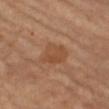workup: catalogued during a skin exam; not biopsied
image: ~15 mm tile from a whole-body skin photo
size: ~3.5 mm (longest diameter)
site: the arm
patient: female, in their 70s
lighting: cross-polarized illumination